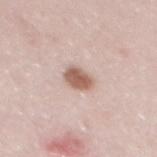Imaged during a routine full-body skin examination; the lesion was not biopsied and no histopathology is available. Measured at roughly 3 mm in maximum diameter. The total-body-photography lesion software estimated an area of roughly 5.5 mm² and a symmetry-axis asymmetry near 0.2. A region of skin cropped from a whole-body photographic capture, roughly 15 mm wide. Imaged with white-light lighting. A male subject, in their mid-20s. The lesion is on the mid back.